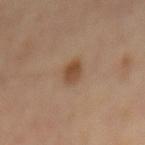| feature | finding |
|---|---|
| follow-up | imaged on a skin check; not biopsied |
| image | total-body-photography crop, ~15 mm field of view |
| body site | the back |